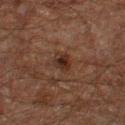Assessment:
Recorded during total-body skin imaging; not selected for excision or biopsy.
Acquisition and patient details:
The lesion-visualizer software estimated a lesion color around L≈21 a*≈15 b*≈19 in CIELAB, about 7 CIELAB-L* units darker than the surrounding skin, and a normalized lesion–skin contrast near 8.5. A roughly 15 mm field-of-view crop from a total-body skin photograph. This is a cross-polarized tile. From the left thigh. The subject is a male in their 60s.